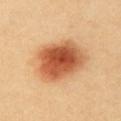Impression: No biopsy was performed on this lesion — it was imaged during a full skin examination and was not determined to be concerning. Context: Automated tile analysis of the lesion measured a lesion area of about 24 mm² and a symmetry-axis asymmetry near 0.15. A close-up tile cropped from a whole-body skin photograph, about 15 mm across. Longest diameter approximately 6 mm. On the chest. The subject is a female in their mid- to late 20s.